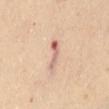Clinical impression: Captured during whole-body skin photography for melanoma surveillance; the lesion was not biopsied. Acquisition and patient details: A female subject approximately 60 years of age. Imaged with cross-polarized lighting. A 15 mm crop from a total-body photograph taken for skin-cancer surveillance. From the abdomen.